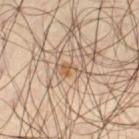This lesion was catalogued during total-body skin photography and was not selected for biopsy.
A male patient aged around 45.
Imaged with cross-polarized lighting.
Automated tile analysis of the lesion measured a shape eccentricity near 0.9 and a shape-asymmetry score of about 0.5 (0 = symmetric). And it measured an average lesion color of about L≈56 a*≈16 b*≈32 (CIELAB), roughly 8 lightness units darker than nearby skin, and a lesion-to-skin contrast of about 6.5 (normalized; higher = more distinct). The software also gave internal color variation of about 1.5 on a 0–10 scale and peripheral color asymmetry of about 0.5. It also reported an automated nevus-likeness rating near 0 out of 100 and a detector confidence of about 10 out of 100 that the crop contains a lesion.
Approximately 3.5 mm at its widest.
A 15 mm close-up tile from a total-body photography series done for melanoma screening.
From the left thigh.